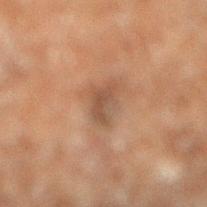Q: Was this lesion biopsied?
A: catalogued during a skin exam; not biopsied
Q: Patient demographics?
A: male, aged 58–62
Q: How was the tile lit?
A: cross-polarized
Q: What did automated image analysis measure?
A: an average lesion color of about L≈38 a*≈15 b*≈25 (CIELAB) and roughly 8 lightness units darker than nearby skin
Q: How was this image acquired?
A: total-body-photography crop, ~15 mm field of view
Q: Lesion location?
A: the right lower leg